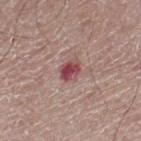Clinical impression: Captured during whole-body skin photography for melanoma surveillance; the lesion was not biopsied. Context: Measured at roughly 2.5 mm in maximum diameter. A male subject about 65 years old. A 15 mm close-up extracted from a 3D total-body photography capture. The lesion-visualizer software estimated an automated nevus-likeness rating near 0 out of 100. From the leg. The tile uses white-light illumination.